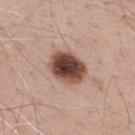| key | value |
|---|---|
| biopsy status | imaged on a skin check; not biopsied |
| anatomic site | the upper back |
| illumination | white-light illumination |
| lesion size | about 4.5 mm |
| subject | male, in their 70s |
| image | ~15 mm tile from a whole-body skin photo |